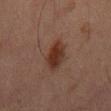Assessment:
The lesion was tiled from a total-body skin photograph and was not biopsied.
Image and clinical context:
Automated image analysis of the tile measured an average lesion color of about L≈25 a*≈17 b*≈22 (CIELAB) and a normalized lesion–skin contrast near 10. The software also gave a border-irregularity rating of about 2/10 and radial color variation of about 1. This is a cross-polarized tile. A roughly 15 mm field-of-view crop from a total-body skin photograph. From the abdomen. Approximately 4 mm at its widest. A male subject aged around 65.